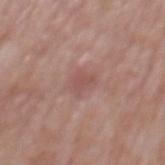Clinical impression:
Captured during whole-body skin photography for melanoma surveillance; the lesion was not biopsied.
Acquisition and patient details:
The lesion's longest dimension is about 2.5 mm. From the mid back. A male subject, roughly 65 years of age. Automated image analysis of the tile measured a lesion area of about 4.5 mm². The software also gave a mean CIELAB color near L≈52 a*≈22 b*≈23, about 7 CIELAB-L* units darker than the surrounding skin, and a normalized lesion–skin contrast near 5. A roughly 15 mm field-of-view crop from a total-body skin photograph. Imaged with white-light lighting.